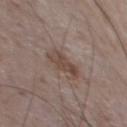Q: Is there a histopathology result?
A: no biopsy performed (imaged during a skin exam)
Q: What did automated image analysis measure?
A: two-axis asymmetry of about 0.3; a lesion–skin lightness drop of about 8 and a normalized lesion–skin contrast near 7; a border-irregularity index near 3.5/10 and internal color variation of about 3.5 on a 0–10 scale
Q: Lesion location?
A: the front of the torso
Q: Illumination type?
A: white-light illumination
Q: What are the patient's age and sex?
A: male, approximately 75 years of age
Q: What is the imaging modality?
A: ~15 mm tile from a whole-body skin photo
Q: Lesion size?
A: ≈3.5 mm